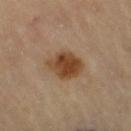Part of a total-body skin-imaging series; this lesion was reviewed on a skin check and was not flagged for biopsy. A female subject, aged around 70. The tile uses cross-polarized illumination. Automated image analysis of the tile measured an area of roughly 10 mm², an outline eccentricity of about 0.65 (0 = round, 1 = elongated), and two-axis asymmetry of about 0.2. It also reported roughly 13 lightness units darker than nearby skin and a lesion-to-skin contrast of about 10.5 (normalized; higher = more distinct). The recorded lesion diameter is about 4 mm. Cropped from a whole-body photographic skin survey; the tile spans about 15 mm. Located on the right thigh.